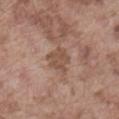The lesion was photographed on a routine skin check and not biopsied; there is no pathology result. Cropped from a total-body skin-imaging series; the visible field is about 15 mm. A male subject, aged approximately 75. The tile uses white-light illumination. The lesion is on the abdomen.